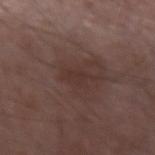Case summary:
- biopsy status: no biopsy performed (imaged during a skin exam)
- subject: male, in their mid-70s
- imaging modality: ~15 mm crop, total-body skin-cancer survey
- body site: the right forearm